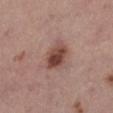Imaged during a routine full-body skin examination; the lesion was not biopsied and no histopathology is available.
Cropped from a total-body skin-imaging series; the visible field is about 15 mm.
The tile uses white-light illumination.
The subject is a female roughly 65 years of age.
Measured at roughly 3.5 mm in maximum diameter.
The lesion is on the left lower leg.
An algorithmic analysis of the crop reported an outline eccentricity of about 0.65 (0 = round, 1 = elongated) and a symmetry-axis asymmetry near 0.25. The analysis additionally found a border-irregularity rating of about 2.5/10, a within-lesion color-variation index near 6/10, and peripheral color asymmetry of about 2. It also reported an automated nevus-likeness rating near 90 out of 100 and a detector confidence of about 100 out of 100 that the crop contains a lesion.